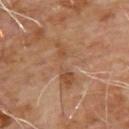Notes:
• tile lighting: cross-polarized illumination
• patient: male, roughly 65 years of age
• imaging modality: ~15 mm tile from a whole-body skin photo
• automated lesion analysis: a border-irregularity index near 7.5/10 and a peripheral color-asymmetry measure near 1; lesion-presence confidence of about 95/100
• body site: the upper back